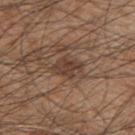Findings:
* notes · imaged on a skin check; not biopsied
* lighting · white-light
* subject · male, about 45 years old
* automated metrics · a classifier nevus-likeness of about 35/100 and lesion-presence confidence of about 95/100
* imaging modality · 15 mm crop, total-body photography
* site · the upper back
* diameter · ~3.5 mm (longest diameter)